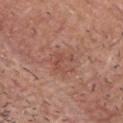Q: Was a biopsy performed?
A: total-body-photography surveillance lesion; no biopsy
Q: Where on the body is the lesion?
A: the head or neck
Q: Who is the patient?
A: male, in their mid-50s
Q: What is the imaging modality?
A: ~15 mm crop, total-body skin-cancer survey